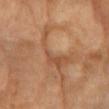Imaged during a routine full-body skin examination; the lesion was not biopsied and no histopathology is available. A region of skin cropped from a whole-body photographic capture, roughly 15 mm wide. A female patient, aged 73 to 77. Imaged with cross-polarized lighting. The lesion is located on the upper back. Measured at roughly 5.5 mm in maximum diameter.